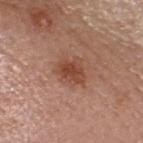site: the head or neck
image: 15 mm crop, total-body photography
subject: female, aged 63 to 67
lighting: white-light illumination